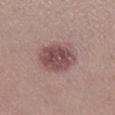Captured during whole-body skin photography for melanoma surveillance; the lesion was not biopsied.
The tile uses white-light illumination.
The lesion is on the right lower leg.
A 15 mm crop from a total-body photograph taken for skin-cancer surveillance.
About 5 mm across.
A female subject aged around 20.
Automated tile analysis of the lesion measured a footprint of about 15 mm², a shape eccentricity near 0.65, and a symmetry-axis asymmetry near 0.15. It also reported a classifier nevus-likeness of about 55/100 and a lesion-detection confidence of about 100/100.